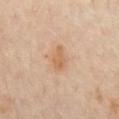| key | value |
|---|---|
| biopsy status | catalogued during a skin exam; not biopsied |
| imaging modality | ~15 mm tile from a whole-body skin photo |
| site | the front of the torso |
| subject | male, aged 63–67 |
| lesion size | about 3.5 mm |
| tile lighting | cross-polarized illumination |